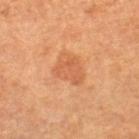The lesion was tiled from a total-body skin photograph and was not biopsied. From the leg. The subject is a female roughly 65 years of age. About 3.5 mm across. The lesion-visualizer software estimated a lesion color around L≈59 a*≈27 b*≈40 in CIELAB, about 8 CIELAB-L* units darker than the surrounding skin, and a normalized lesion–skin contrast near 5.5. And it measured border irregularity of about 3.5 on a 0–10 scale and radial color variation of about 1. The software also gave a nevus-likeness score of about 15/100 and a detector confidence of about 100 out of 100 that the crop contains a lesion. A 15 mm crop from a total-body photograph taken for skin-cancer surveillance. The tile uses cross-polarized illumination.